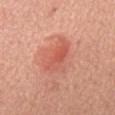biopsy_status: not biopsied; imaged during a skin examination
lighting: white-light
image:
  source: total-body photography crop
  field_of_view_mm: 15
lesion_size:
  long_diameter_mm_approx: 5.5
patient:
  sex: male
  age_approx: 65
site: chest